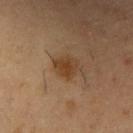Assessment:
Captured during whole-body skin photography for melanoma surveillance; the lesion was not biopsied.